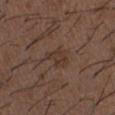Part of a total-body skin-imaging series; this lesion was reviewed on a skin check and was not flagged for biopsy. A male subject, approximately 50 years of age. Approximately 3 mm at its widest. On the chest. A close-up tile cropped from a whole-body skin photograph, about 15 mm across. This is a white-light tile.